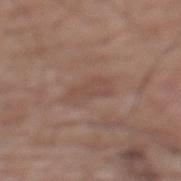Part of a total-body skin-imaging series; this lesion was reviewed on a skin check and was not flagged for biopsy.
A male subject, roughly 60 years of age.
The lesion's longest dimension is about 4 mm.
A close-up tile cropped from a whole-body skin photograph, about 15 mm across.
The lesion is located on the mid back.
The tile uses white-light illumination.